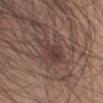{"biopsy_status": "not biopsied; imaged during a skin examination", "lesion_size": {"long_diameter_mm_approx": 4.5}, "site": "right upper arm", "patient": {"sex": "male", "age_approx": 20}, "lighting": "white-light", "automated_metrics": {"area_mm2_approx": 9.0, "eccentricity": 0.8, "cielab_L": 39, "cielab_a": 17, "cielab_b": 21, "vs_skin_contrast_norm": 6.5}, "image": {"source": "total-body photography crop", "field_of_view_mm": 15}}